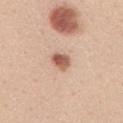This image is a 15 mm lesion crop taken from a total-body photograph. An algorithmic analysis of the crop reported a footprint of about 4.5 mm², an outline eccentricity of about 0.65 (0 = round, 1 = elongated), and two-axis asymmetry of about 0.25. And it measured internal color variation of about 5 on a 0–10 scale. This is a white-light tile. On the mid back. Measured at roughly 2.5 mm in maximum diameter. The patient is a female approximately 45 years of age.